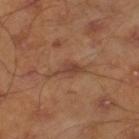Assessment: Part of a total-body skin-imaging series; this lesion was reviewed on a skin check and was not flagged for biopsy.